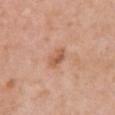Q: Was this lesion biopsied?
A: total-body-photography surveillance lesion; no biopsy
Q: How large is the lesion?
A: ~3 mm (longest diameter)
Q: Where on the body is the lesion?
A: the chest
Q: What is the imaging modality?
A: total-body-photography crop, ~15 mm field of view
Q: Illumination type?
A: white-light
Q: Who is the patient?
A: female, aged 68–72